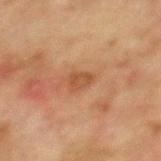automated lesion analysis: an eccentricity of roughly 0.8 and a symmetry-axis asymmetry near 0.3; a lesion color around L≈42 a*≈20 b*≈31 in CIELAB, a lesion–skin lightness drop of about 8, and a lesion-to-skin contrast of about 6.5 (normalized; higher = more distinct); an automated nevus-likeness rating near 0 out of 100
lesion size: ≈2.5 mm
acquisition: 15 mm crop, total-body photography
illumination: cross-polarized illumination
site: the mid back
subject: male, aged 73–77